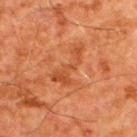biopsy status = imaged on a skin check; not biopsied | lesion diameter = about 5 mm | patient = male, aged approximately 60 | anatomic site = the upper back | image source = ~15 mm tile from a whole-body skin photo.